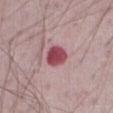Automated tile analysis of the lesion measured a shape eccentricity near 0.4.
On the abdomen.
A close-up tile cropped from a whole-body skin photograph, about 15 mm across.
A male patient, aged around 75.
This is a white-light tile.
Approximately 3 mm at its widest.